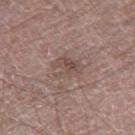{"biopsy_status": "not biopsied; imaged during a skin examination", "automated_metrics": {"vs_skin_darker_L": 7.0, "vs_skin_contrast_norm": 5.5, "nevus_likeness_0_100": 0, "lesion_detection_confidence_0_100": 100}, "lighting": "white-light", "site": "right thigh", "patient": {"sex": "male", "age_approx": 65}, "image": {"source": "total-body photography crop", "field_of_view_mm": 15}, "lesion_size": {"long_diameter_mm_approx": 3.5}}